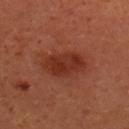This lesion was catalogued during total-body skin photography and was not selected for biopsy.
Measured at roughly 5 mm in maximum diameter.
This is a cross-polarized tile.
Automated image analysis of the tile measured an eccentricity of roughly 0.85. The analysis additionally found roughly 9 lightness units darker than nearby skin. The software also gave an automated nevus-likeness rating near 80 out of 100 and lesion-presence confidence of about 100/100.
A roughly 15 mm field-of-view crop from a total-body skin photograph.
The lesion is on the back.
A female patient, aged 38 to 42.